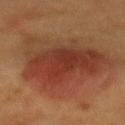Assessment: No biopsy was performed on this lesion — it was imaged during a full skin examination and was not determined to be concerning. Acquisition and patient details: This is a cross-polarized tile. The lesion-visualizer software estimated a shape eccentricity near 0.5. The analysis additionally found about 8 CIELAB-L* units darker than the surrounding skin and a lesion-to-skin contrast of about 8 (normalized; higher = more distinct). Longest diameter approximately 9.5 mm. A close-up tile cropped from a whole-body skin photograph, about 15 mm across. The subject is a female in their 50s. From the mid back.